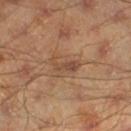follow-up: total-body-photography surveillance lesion; no biopsy | acquisition: total-body-photography crop, ~15 mm field of view | patient: male, in their mid-40s | location: the left lower leg.